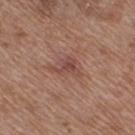  site: right thigh
  lighting: white-light
  lesion_size:
    long_diameter_mm_approx: 3.5
  image:
    source: total-body photography crop
    field_of_view_mm: 15
  patient:
    sex: female
    age_approx: 75
  automated_metrics:
    cielab_L: 46
    cielab_a: 22
    cielab_b: 24
    vs_skin_darker_L: 8.0
    nevus_likeness_0_100: 0
    lesion_detection_confidence_0_100: 100